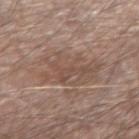Impression: Part of a total-body skin-imaging series; this lesion was reviewed on a skin check and was not flagged for biopsy. Clinical summary: Measured at roughly 7 mm in maximum diameter. Located on the left forearm. Cropped from a total-body skin-imaging series; the visible field is about 15 mm. The subject is a male approximately 60 years of age. The total-body-photography lesion software estimated a footprint of about 21 mm², an eccentricity of roughly 0.8, and a symmetry-axis asymmetry near 0.25. The analysis additionally found a lesion color around L≈50 a*≈16 b*≈25 in CIELAB and about 7 CIELAB-L* units darker than the surrounding skin.